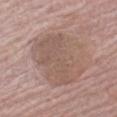{"biopsy_status": "not biopsied; imaged during a skin examination", "lighting": "white-light", "patient": {"sex": "male", "age_approx": 65}, "image": {"source": "total-body photography crop", "field_of_view_mm": 15}, "site": "left upper arm"}